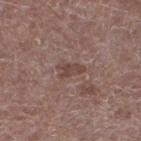{"biopsy_status": "not biopsied; imaged during a skin examination", "lighting": "white-light", "image": {"source": "total-body photography crop", "field_of_view_mm": 15}, "patient": {"sex": "male", "age_approx": 70}, "site": "leg"}